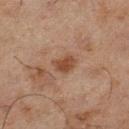Impression: Imaged during a routine full-body skin examination; the lesion was not biopsied and no histopathology is available. Clinical summary: The subject is a male in their mid- to late 40s. On the left lower leg. The lesion-visualizer software estimated an average lesion color of about L≈37 a*≈18 b*≈26 (CIELAB) and about 8 CIELAB-L* units darker than the surrounding skin. It also reported a border-irregularity index near 2.5/10, a color-variation rating of about 2/10, and radial color variation of about 0.5. Captured under cross-polarized illumination. About 2.5 mm across. A 15 mm close-up tile from a total-body photography series done for melanoma screening.